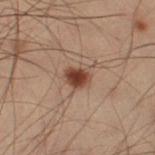notes: imaged on a skin check; not biopsied
automated lesion analysis: a footprint of about 4.5 mm², an outline eccentricity of about 0.6 (0 = round, 1 = elongated), and two-axis asymmetry of about 0.25
imaging modality: total-body-photography crop, ~15 mm field of view
diameter: ≈2.5 mm
site: the right thigh
patient: male, about 55 years old